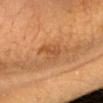{"biopsy_status": "not biopsied; imaged during a skin examination", "patient": {"sex": "male", "age_approx": "85+"}, "image": {"source": "total-body photography crop", "field_of_view_mm": 15}, "automated_metrics": {"cielab_L": 44, "cielab_a": 22, "cielab_b": 36, "vs_skin_darker_L": 6.0, "nevus_likeness_0_100": 15, "lesion_detection_confidence_0_100": 100}, "site": "head or neck", "lesion_size": {"long_diameter_mm_approx": 2.5}}